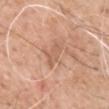Notes:
– location: the left upper arm
– subject: male, approximately 60 years of age
– acquisition: 15 mm crop, total-body photography
– lighting: white-light illumination